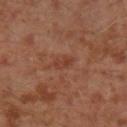illumination = cross-polarized | site = the left lower leg | subject = male, in their 30s | image source = ~15 mm tile from a whole-body skin photo | automated metrics = an area of roughly 3 mm² and a symmetry-axis asymmetry near 0.25; border irregularity of about 2.5 on a 0–10 scale, a within-lesion color-variation index near 0.5/10, and radial color variation of about 0.